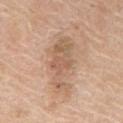Case summary:
- workup · total-body-photography surveillance lesion; no biopsy
- location · the chest
- acquisition · 15 mm crop, total-body photography
- patient · male, about 65 years old
- lesion size · ≈7 mm
- tile lighting · white-light illumination